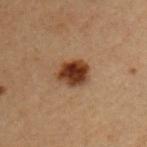<record>
<biopsy_status>not biopsied; imaged during a skin examination</biopsy_status>
<site>chest</site>
<automated_metrics>
  <area_mm2_approx>8.5</area_mm2_approx>
  <eccentricity>0.65</eccentricity>
  <shape_asymmetry>0.2</shape_asymmetry>
  <cielab_L>29</cielab_L>
  <cielab_a>18</cielab_a>
  <cielab_b>26</cielab_b>
  <vs_skin_darker_L>14.0</vs_skin_darker_L>
  <vs_skin_contrast_norm>13.0</vs_skin_contrast_norm>
  <border_irregularity_0_10>2.0</border_irregularity_0_10>
  <peripheral_color_asymmetry>1.5</peripheral_color_asymmetry>
  <nevus_likeness_0_100>100</nevus_likeness_0_100>
  <lesion_detection_confidence_0_100>100</lesion_detection_confidence_0_100>
</automated_metrics>
<patient>
  <sex>male</sex>
  <age_approx>50</age_approx>
</patient>
<lesion_size>
  <long_diameter_mm_approx>4.0</long_diameter_mm_approx>
</lesion_size>
<lighting>cross-polarized</lighting>
<image>
  <source>total-body photography crop</source>
  <field_of_view_mm>15</field_of_view_mm>
</image>
</record>